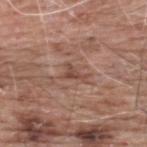Notes:
- patient — male, roughly 60 years of age
- image source — total-body-photography crop, ~15 mm field of view
- image-analysis metrics — an area of roughly 3 mm² and a shape eccentricity near 0.85; a mean CIELAB color near L≈47 a*≈21 b*≈26, roughly 9 lightness units darker than nearby skin, and a normalized border contrast of about 7; a border-irregularity index near 6.5/10, a within-lesion color-variation index near 0.5/10, and a peripheral color-asymmetry measure near 0; a classifier nevus-likeness of about 0/100 and a lesion-detection confidence of about 90/100
- lesion size — ≈2.5 mm
- site — the upper back
- tile lighting — white-light